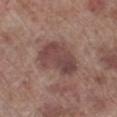Clinical summary:
A 15 mm close-up tile from a total-body photography series done for melanoma screening. The tile uses white-light illumination. The lesion is on the left lower leg. The patient is a male aged around 70. The recorded lesion diameter is about 5.5 mm.